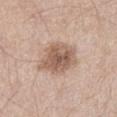follow-up: no biopsy performed (imaged during a skin exam).